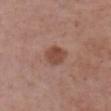Case summary:
– lesion diameter: ≈3 mm
– tile lighting: white-light illumination
– image source: ~15 mm crop, total-body skin-cancer survey
– automated lesion analysis: an area of roughly 6.5 mm² and a shape eccentricity near 0.5; a mean CIELAB color near L≈47 a*≈22 b*≈27 and roughly 10 lightness units darker than nearby skin; a border-irregularity index near 2/10, internal color variation of about 2 on a 0–10 scale, and peripheral color asymmetry of about 1; a nevus-likeness score of about 85/100 and lesion-presence confidence of about 100/100
– body site: the chest
– subject: female, aged 73 to 77